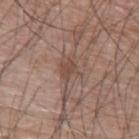workup=total-body-photography surveillance lesion; no biopsy | lighting=white-light | image=total-body-photography crop, ~15 mm field of view | site=the arm | subject=male, aged approximately 75.